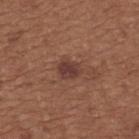Q: Illumination type?
A: white-light illumination
Q: What is the lesion's diameter?
A: about 3 mm
Q: Where on the body is the lesion?
A: the upper back
Q: What did automated image analysis measure?
A: an area of roughly 5 mm² and a shape eccentricity near 0.55; a lesion color around L≈38 a*≈20 b*≈23 in CIELAB, a lesion–skin lightness drop of about 9, and a normalized border contrast of about 8.5
Q: Patient demographics?
A: female, aged 63 to 67
Q: What is the imaging modality?
A: ~15 mm tile from a whole-body skin photo